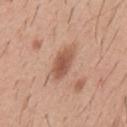Part of a total-body skin-imaging series; this lesion was reviewed on a skin check and was not flagged for biopsy. A male patient aged 38–42. The lesion is located on the mid back. Captured under white-light illumination. A roughly 15 mm field-of-view crop from a total-body skin photograph. Approximately 4 mm at its widest. Automated image analysis of the tile measured border irregularity of about 2 on a 0–10 scale, a within-lesion color-variation index near 3/10, and peripheral color asymmetry of about 1. And it measured a classifier nevus-likeness of about 80/100 and lesion-presence confidence of about 100/100.